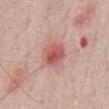| field | value |
|---|---|
| subject | male, in their 70s |
| automated metrics | a footprint of about 8 mm², an eccentricity of roughly 0.6, and two-axis asymmetry of about 0.2; a lesion color around L≈56 a*≈30 b*≈23 in CIELAB, about 11 CIELAB-L* units darker than the surrounding skin, and a lesion-to-skin contrast of about 7.5 (normalized; higher = more distinct); border irregularity of about 2 on a 0–10 scale, a color-variation rating of about 6/10, and a peripheral color-asymmetry measure near 1.5; a classifier nevus-likeness of about 5/100 and lesion-presence confidence of about 100/100 |
| lesion diameter | about 3.5 mm |
| imaging modality | ~15 mm crop, total-body skin-cancer survey |
| lighting | white-light illumination |
| anatomic site | the chest |